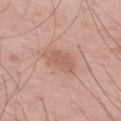Imaged during a routine full-body skin examination; the lesion was not biopsied and no histopathology is available.
A male subject, about 55 years old.
From the left thigh.
Cropped from a whole-body photographic skin survey; the tile spans about 15 mm.
An algorithmic analysis of the crop reported a lesion area of about 9.5 mm², an eccentricity of roughly 0.85, and a symmetry-axis asymmetry near 0.25. The analysis additionally found a lesion color around L≈59 a*≈22 b*≈27 in CIELAB, roughly 8 lightness units darker than nearby skin, and a normalized lesion–skin contrast near 5. The software also gave a border-irregularity rating of about 3/10, a color-variation rating of about 2/10, and a peripheral color-asymmetry measure near 1. It also reported lesion-presence confidence of about 100/100.
The lesion's longest dimension is about 5 mm.